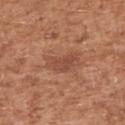{"biopsy_status": "not biopsied; imaged during a skin examination", "automated_metrics": {"area_mm2_approx": 6.0, "eccentricity": 0.85, "shape_asymmetry": 0.35, "nevus_likeness_0_100": 0, "lesion_detection_confidence_0_100": 100}, "lighting": "white-light", "site": "right upper arm", "patient": {"sex": "male", "age_approx": 45}, "image": {"source": "total-body photography crop", "field_of_view_mm": 15}}